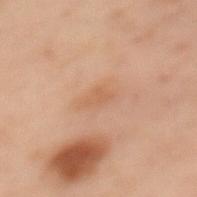{"lesion_size": {"long_diameter_mm_approx": 2.5}, "lighting": "cross-polarized", "patient": {"sex": "female", "age_approx": 55}, "site": "mid back", "image": {"source": "total-body photography crop", "field_of_view_mm": 15}}